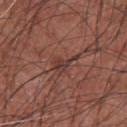<lesion>
<patient>
  <sex>male</sex>
  <age_approx>75</age_approx>
</patient>
<lighting>white-light</lighting>
<site>chest</site>
<image>
  <source>total-body photography crop</source>
  <field_of_view_mm>15</field_of_view_mm>
</image>
<automated_metrics>
  <cielab_L>37</cielab_L>
  <cielab_a>21</cielab_a>
  <cielab_b>23</cielab_b>
  <vs_skin_darker_L>8.0</vs_skin_darker_L>
  <vs_skin_contrast_norm>7.0</vs_skin_contrast_norm>
  <border_irregularity_0_10>6.5</border_irregularity_0_10>
  <color_variation_0_10>4.0</color_variation_0_10>
  <peripheral_color_asymmetry>1.5</peripheral_color_asymmetry>
</automated_metrics>
<lesion_size>
  <long_diameter_mm_approx>3.5</long_diameter_mm_approx>
</lesion_size>
</lesion>